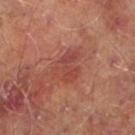biopsy status: imaged on a skin check; not biopsied.